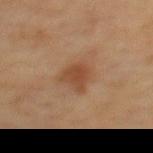biopsy_status: not biopsied; imaged during a skin examination
patient:
  sex: female
  age_approx: 55
lesion_size:
  long_diameter_mm_approx: 3.5
image:
  source: total-body photography crop
  field_of_view_mm: 15
site: back
automated_metrics:
  area_mm2_approx: 7.0
  eccentricity: 0.7
  shape_asymmetry: 0.3
  border_irregularity_0_10: 3.5
  color_variation_0_10: 2.5
  peripheral_color_asymmetry: 1.0
lighting: cross-polarized